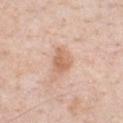notes: total-body-photography surveillance lesion; no biopsy
location: the chest
patient: male, roughly 50 years of age
lighting: white-light illumination
imaging modality: total-body-photography crop, ~15 mm field of view
automated metrics: roughly 10 lightness units darker than nearby skin
lesion diameter: ≈3.5 mm